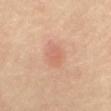notes=no biopsy performed (imaged during a skin exam)
image source=~15 mm crop, total-body skin-cancer survey
size=≈2.5 mm
subject=female, in their 70s
location=the mid back
tile lighting=cross-polarized illumination